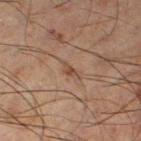{"biopsy_status": "not biopsied; imaged during a skin examination", "image": {"source": "total-body photography crop", "field_of_view_mm": 15}, "patient": {"sex": "male", "age_approx": 65}, "lighting": "cross-polarized", "site": "right thigh"}